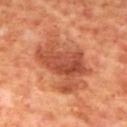workup = total-body-photography surveillance lesion; no biopsy
site = the mid back
lesion size = ~7.5 mm (longest diameter)
illumination = cross-polarized
patient = male, aged 68 to 72
acquisition = ~15 mm tile from a whole-body skin photo
image-analysis metrics = a lesion area of about 29 mm²